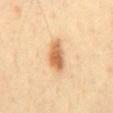Captured during whole-body skin photography for melanoma surveillance; the lesion was not biopsied.
This is a cross-polarized tile.
On the abdomen.
The subject is a male aged around 40.
Longest diameter approximately 4 mm.
A region of skin cropped from a whole-body photographic capture, roughly 15 mm wide.